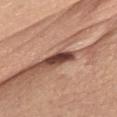Captured during whole-body skin photography for melanoma surveillance; the lesion was not biopsied. A 15 mm close-up extracted from a 3D total-body photography capture. A female patient in their mid-50s. Captured under white-light illumination. The lesion's longest dimension is about 3 mm. The lesion is located on the chest.